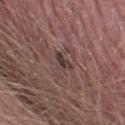No biopsy was performed on this lesion — it was imaged during a full skin examination and was not determined to be concerning.
A roughly 15 mm field-of-view crop from a total-body skin photograph.
Captured under white-light illumination.
Located on the left thigh.
A male patient in their 50s.
Longest diameter approximately 3 mm.